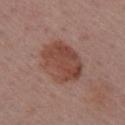<tbp_lesion>
<biopsy_status>not biopsied; imaged during a skin examination</biopsy_status>
<lighting>white-light</lighting>
<site>arm</site>
<patient>
  <sex>female</sex>
  <age_approx>55</age_approx>
</patient>
<image>
  <source>total-body photography crop</source>
  <field_of_view_mm>15</field_of_view_mm>
</image>
</tbp_lesion>